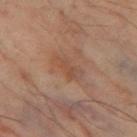notes: catalogued during a skin exam; not biopsied | patient: male, aged 63–67 | body site: the left thigh | diameter: ≈4 mm | lighting: cross-polarized illumination | acquisition: total-body-photography crop, ~15 mm field of view.